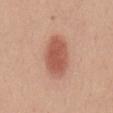The lesion was tiled from a total-body skin photograph and was not biopsied. The patient is a female roughly 50 years of age. From the mid back. A 15 mm close-up extracted from a 3D total-body photography capture.